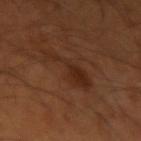| key | value |
|---|---|
| follow-up | imaged on a skin check; not biopsied |
| lighting | cross-polarized illumination |
| anatomic site | the left upper arm |
| size | about 6 mm |
| subject | male, approximately 55 years of age |
| image | ~15 mm tile from a whole-body skin photo |